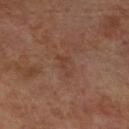follow-up: no biopsy performed (imaged during a skin exam)
body site: the right lower leg
lesion size: about 3 mm
patient: male, aged around 70
lighting: cross-polarized illumination
acquisition: total-body-photography crop, ~15 mm field of view
TBP lesion metrics: an area of roughly 2.5 mm², an outline eccentricity of about 0.9 (0 = round, 1 = elongated), and a shape-asymmetry score of about 0.65 (0 = symmetric); a lesion color around L≈38 a*≈20 b*≈26 in CIELAB, roughly 5 lightness units darker than nearby skin, and a normalized lesion–skin contrast near 4.5; border irregularity of about 7 on a 0–10 scale, internal color variation of about 0 on a 0–10 scale, and radial color variation of about 0; an automated nevus-likeness rating near 0 out of 100 and lesion-presence confidence of about 100/100